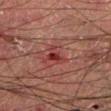Q: Lesion location?
A: the leg
Q: Patient demographics?
A: male, aged 53 to 57
Q: What is the lesion's diameter?
A: ≈3 mm
Q: How was the tile lit?
A: cross-polarized illumination
Q: What kind of image is this?
A: ~15 mm crop, total-body skin-cancer survey